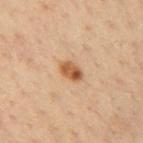Imaged with cross-polarized lighting.
Cropped from a total-body skin-imaging series; the visible field is about 15 mm.
An algorithmic analysis of the crop reported roughly 12 lightness units darker than nearby skin and a normalized border contrast of about 9.5. The analysis additionally found a border-irregularity rating of about 1.5/10, internal color variation of about 6.5 on a 0–10 scale, and peripheral color asymmetry of about 2.5.
Longest diameter approximately 3 mm.
A male subject aged around 35.
The lesion is on the left upper arm.